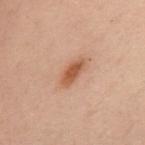{
  "biopsy_status": "not biopsied; imaged during a skin examination",
  "lesion_size": {
    "long_diameter_mm_approx": 3.5
  },
  "lighting": "cross-polarized",
  "automated_metrics": {
    "area_mm2_approx": 5.0,
    "eccentricity": 0.9,
    "shape_asymmetry": 0.15
  },
  "site": "back",
  "image": {
    "source": "total-body photography crop",
    "field_of_view_mm": 15
  },
  "patient": {
    "sex": "female",
    "age_approx": 55
  }
}